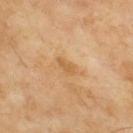workup=catalogued during a skin exam; not biopsied.